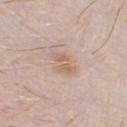Clinical impression: The lesion was tiled from a total-body skin photograph and was not biopsied. Background: The lesion is on the chest. Automated image analysis of the tile measured a shape eccentricity near 0.8. The analysis additionally found an average lesion color of about L≈63 a*≈16 b*≈29 (CIELAB), roughly 7 lightness units darker than nearby skin, and a lesion-to-skin contrast of about 6.5 (normalized; higher = more distinct). The software also gave a peripheral color-asymmetry measure near 1. A male subject, approximately 30 years of age. Cropped from a total-body skin-imaging series; the visible field is about 15 mm. About 3.5 mm across. This is a white-light tile.